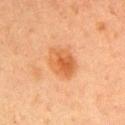biopsy status = total-body-photography surveillance lesion; no biopsy | patient = female, aged approximately 60 | image = ~15 mm tile from a whole-body skin photo | location = the arm.